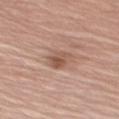The lesion was photographed on a routine skin check and not biopsied; there is no pathology result. From the right upper arm. A lesion tile, about 15 mm wide, cut from a 3D total-body photograph. A male subject roughly 75 years of age.